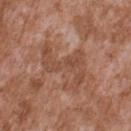{"biopsy_status": "not biopsied; imaged during a skin examination", "image": {"source": "total-body photography crop", "field_of_view_mm": 15}, "patient": {"sex": "male", "age_approx": 45}, "site": "upper back"}